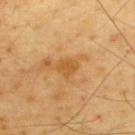| key | value |
|---|---|
| notes | catalogued during a skin exam; not biopsied |
| imaging modality | total-body-photography crop, ~15 mm field of view |
| patient | male, in their mid- to late 60s |
| lighting | cross-polarized illumination |
| location | the upper back |
| lesion diameter | ~2.5 mm (longest diameter) |